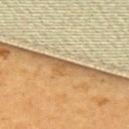follow-up — total-body-photography surveillance lesion; no biopsy | diameter — about 2 mm | site — the upper back | subject — female, aged around 55 | image source — total-body-photography crop, ~15 mm field of view | illumination — cross-polarized.